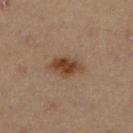Impression:
The lesion was tiled from a total-body skin photograph and was not biopsied.
Context:
Captured under cross-polarized illumination. On the leg. A roughly 15 mm field-of-view crop from a total-body skin photograph. Measured at roughly 4 mm in maximum diameter. The total-body-photography lesion software estimated an outline eccentricity of about 0.8 (0 = round, 1 = elongated) and a symmetry-axis asymmetry near 0.2. The analysis additionally found internal color variation of about 4 on a 0–10 scale and a peripheral color-asymmetry measure near 1. The software also gave a nevus-likeness score of about 95/100 and a detector confidence of about 100 out of 100 that the crop contains a lesion. A female patient, about 45 years old.